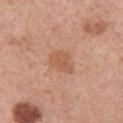Q: Was this lesion biopsied?
A: total-body-photography surveillance lesion; no biopsy
Q: What is the anatomic site?
A: the right upper arm
Q: What is the lesion's diameter?
A: ≈3.5 mm
Q: What is the imaging modality?
A: 15 mm crop, total-body photography
Q: What are the patient's age and sex?
A: female, aged 58–62
Q: How was the tile lit?
A: white-light illumination